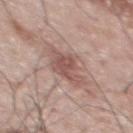Imaged during a routine full-body skin examination; the lesion was not biopsied and no histopathology is available.
A 15 mm close-up extracted from a 3D total-body photography capture.
The lesion is on the upper back.
Imaged with white-light lighting.
The subject is a male roughly 55 years of age.
The recorded lesion diameter is about 4.5 mm.
The total-body-photography lesion software estimated a lesion color around L≈54 a*≈20 b*≈23 in CIELAB, about 10 CIELAB-L* units darker than the surrounding skin, and a normalized lesion–skin contrast near 6.5. It also reported a border-irregularity rating of about 3.5/10. The analysis additionally found lesion-presence confidence of about 100/100.